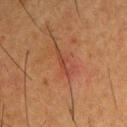Findings:
- imaging modality · ~15 mm tile from a whole-body skin photo
- diameter · ~4 mm (longest diameter)
- subject · male, approximately 35 years of age
- illumination · cross-polarized illumination
- site · the upper back